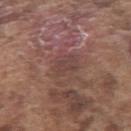No biopsy was performed on this lesion — it was imaged during a full skin examination and was not determined to be concerning. Automated tile analysis of the lesion measured a lesion color around L≈42 a*≈19 b*≈21 in CIELAB, about 6 CIELAB-L* units darker than the surrounding skin, and a normalized border contrast of about 5. The lesion is located on the left upper arm. Captured under white-light illumination. About 3 mm across. A male patient, about 75 years old. A 15 mm crop from a total-body photograph taken for skin-cancer surveillance.